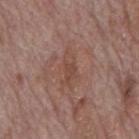Captured during whole-body skin photography for melanoma surveillance; the lesion was not biopsied. On the mid back. A 15 mm crop from a total-body photograph taken for skin-cancer surveillance. A male subject, approximately 70 years of age.